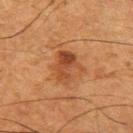The lesion was photographed on a routine skin check and not biopsied; there is no pathology result.
A region of skin cropped from a whole-body photographic capture, roughly 15 mm wide.
The tile uses cross-polarized illumination.
Longest diameter approximately 4.5 mm.
A male subject, aged 48–52.
The total-body-photography lesion software estimated a lesion area of about 10 mm², an outline eccentricity of about 0.65 (0 = round, 1 = elongated), and a shape-asymmetry score of about 0.3 (0 = symmetric).
The lesion is located on the left upper arm.